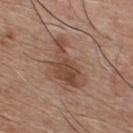follow-up = imaged on a skin check; not biopsied | automated metrics = an average lesion color of about L≈46 a*≈20 b*≈28 (CIELAB) and a normalized lesion–skin contrast near 8; a classifier nevus-likeness of about 30/100 and lesion-presence confidence of about 100/100 | diameter = about 6.5 mm | illumination = white-light illumination | site = the chest | image source = 15 mm crop, total-body photography | subject = male, aged around 75.